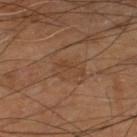| field | value |
|---|---|
| biopsy status | no biopsy performed (imaged during a skin exam) |
| subject | male, approximately 70 years of age |
| acquisition | total-body-photography crop, ~15 mm field of view |
| body site | the right lower leg |